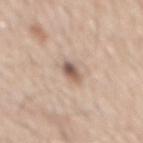* biopsy status — total-body-photography surveillance lesion; no biopsy
* site — the back
* subject — male, in their mid-60s
* image-analysis metrics — a footprint of about 4 mm², a shape eccentricity near 0.75, and a shape-asymmetry score of about 0.15 (0 = symmetric); a lesion color around L≈58 a*≈16 b*≈25 in CIELAB and roughly 14 lightness units darker than nearby skin
* image — total-body-photography crop, ~15 mm field of view
* tile lighting — white-light illumination